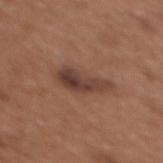| feature | finding |
|---|---|
| notes | imaged on a skin check; not biopsied |
| patient | female, approximately 35 years of age |
| lesion diameter | ≈5.5 mm |
| image-analysis metrics | a lesion area of about 9.5 mm², a shape eccentricity near 0.95, and a symmetry-axis asymmetry near 0.3; a lesion color around L≈40 a*≈19 b*≈25 in CIELAB, about 10 CIELAB-L* units darker than the surrounding skin, and a normalized border contrast of about 9 |
| illumination | white-light illumination |
| acquisition | 15 mm crop, total-body photography |
| body site | the chest |